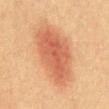workup: no biopsy performed (imaged during a skin exam) | automated lesion analysis: a lesion area of about 28 mm² and an outline eccentricity of about 0.85 (0 = round, 1 = elongated); a mean CIELAB color near L≈49 a*≈23 b*≈31, a lesion–skin lightness drop of about 10, and a lesion-to-skin contrast of about 7.5 (normalized; higher = more distinct); border irregularity of about 2.5 on a 0–10 scale and a color-variation rating of about 3.5/10 | diameter: ~8.5 mm (longest diameter) | lighting: cross-polarized illumination | anatomic site: the abdomen | patient: male, aged 38 to 42 | image: 15 mm crop, total-body photography.